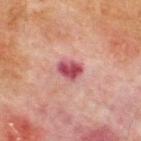Recorded during total-body skin imaging; not selected for excision or biopsy.